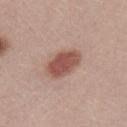{"lesion_size": {"long_diameter_mm_approx": 4.5}, "image": {"source": "total-body photography crop", "field_of_view_mm": 15}, "patient": {"sex": "male", "age_approx": 30}, "lighting": "white-light"}